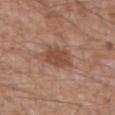Q: Is there a histopathology result?
A: total-body-photography surveillance lesion; no biopsy
Q: What is the imaging modality?
A: 15 mm crop, total-body photography
Q: How large is the lesion?
A: ≈3.5 mm
Q: What lighting was used for the tile?
A: white-light illumination
Q: Patient demographics?
A: male, in their 60s
Q: Lesion location?
A: the mid back